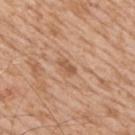Q: Was this lesion biopsied?
A: no biopsy performed (imaged during a skin exam)
Q: What are the patient's age and sex?
A: male, about 65 years old
Q: What kind of image is this?
A: ~15 mm crop, total-body skin-cancer survey
Q: What lighting was used for the tile?
A: white-light
Q: Lesion location?
A: the right upper arm
Q: Lesion size?
A: ≈2.5 mm